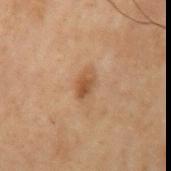anatomic site = the left upper arm | subject = male, aged around 70 | image source = total-body-photography crop, ~15 mm field of view | lesion diameter = ~3 mm (longest diameter).